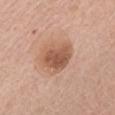| field | value |
|---|---|
| biopsy status | imaged on a skin check; not biopsied |
| subject | female, in their mid-70s |
| lesion size | ≈4 mm |
| site | the mid back |
| lighting | white-light illumination |
| acquisition | ~15 mm tile from a whole-body skin photo |